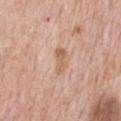Notes:
– biopsy status: total-body-photography surveillance lesion; no biopsy
– tile lighting: white-light illumination
– subject: male, about 60 years old
– image: total-body-photography crop, ~15 mm field of view
– body site: the chest
– size: about 3 mm